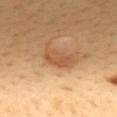* biopsy status · total-body-photography surveillance lesion; no biopsy
* image · ~15 mm crop, total-body skin-cancer survey
* lighting · cross-polarized
* site · the back
* subject · female, aged 48 to 52
* automated metrics · a footprint of about 6 mm² and an outline eccentricity of about 0.9 (0 = round, 1 = elongated)
* size · about 4 mm